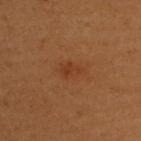No biopsy was performed on this lesion — it was imaged during a full skin examination and was not determined to be concerning.
Longest diameter approximately 3 mm.
Automated tile analysis of the lesion measured an area of roughly 4 mm², an eccentricity of roughly 0.8, and two-axis asymmetry of about 0.15. The software also gave a lesion color around L≈32 a*≈21 b*≈30 in CIELAB, a lesion–skin lightness drop of about 5, and a normalized lesion–skin contrast near 5. The analysis additionally found a border-irregularity rating of about 1.5/10, internal color variation of about 2 on a 0–10 scale, and peripheral color asymmetry of about 1.
The lesion is located on the upper back.
Imaged with cross-polarized lighting.
A female patient, in their 50s.
A 15 mm crop from a total-body photograph taken for skin-cancer surveillance.